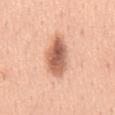Assessment:
Recorded during total-body skin imaging; not selected for excision or biopsy.
Background:
The lesion is on the mid back. A region of skin cropped from a whole-body photographic capture, roughly 15 mm wide. The patient is a male aged around 40. The tile uses white-light illumination. The lesion's longest dimension is about 6 mm.